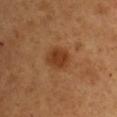The lesion was tiled from a total-body skin photograph and was not biopsied. A 15 mm close-up tile from a total-body photography series done for melanoma screening. The subject is a male aged 48–52. The lesion is on the left upper arm. Imaged with cross-polarized lighting. The recorded lesion diameter is about 3 mm. Automated image analysis of the tile measured an area of roughly 7.5 mm² and two-axis asymmetry of about 0.15. It also reported a lesion color around L≈39 a*≈24 b*≈36 in CIELAB, roughly 9 lightness units darker than nearby skin, and a normalized lesion–skin contrast near 8. It also reported border irregularity of about 1.5 on a 0–10 scale and peripheral color asymmetry of about 1.